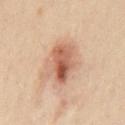The lesion was tiled from a total-body skin photograph and was not biopsied.